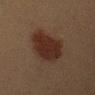Assessment:
Part of a total-body skin-imaging series; this lesion was reviewed on a skin check and was not flagged for biopsy.
Image and clinical context:
A 15 mm crop from a total-body photograph taken for skin-cancer surveillance. Located on the arm. A female patient aged 38–42.